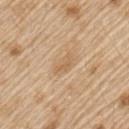Part of a total-body skin-imaging series; this lesion was reviewed on a skin check and was not flagged for biopsy. Longest diameter approximately 3.5 mm. The patient is a male aged approximately 70. From the left upper arm. The total-body-photography lesion software estimated a border-irregularity rating of about 2.5/10, a within-lesion color-variation index near 2/10, and peripheral color asymmetry of about 0.5. This is a white-light tile. A 15 mm crop from a total-body photograph taken for skin-cancer surveillance.